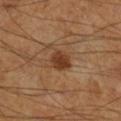Findings:
• biopsy status · no biopsy performed (imaged during a skin exam)
• body site · the right lower leg
• lesion size · ≈3 mm
• image · ~15 mm crop, total-body skin-cancer survey
• patient · male, about 60 years old